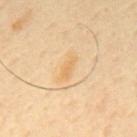Captured during whole-body skin photography for melanoma surveillance; the lesion was not biopsied. Imaged with cross-polarized lighting. The patient is a male in their 60s. Approximately 3 mm at its widest. The lesion is on the mid back. A 15 mm crop from a total-body photograph taken for skin-cancer surveillance.